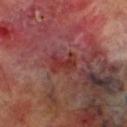The lesion was photographed on a routine skin check and not biopsied; there is no pathology result.
The lesion's longest dimension is about 3 mm.
A 15 mm close-up extracted from a 3D total-body photography capture.
A male patient, aged 68 to 72.
From the right lower leg.
The tile uses cross-polarized illumination.